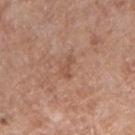Part of a total-body skin-imaging series; this lesion was reviewed on a skin check and was not flagged for biopsy. A region of skin cropped from a whole-body photographic capture, roughly 15 mm wide. Automated tile analysis of the lesion measured a classifier nevus-likeness of about 0/100 and a lesion-detection confidence of about 100/100. A male subject, approximately 60 years of age. From the arm.